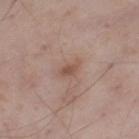Q: How was this image acquired?
A: total-body-photography crop, ~15 mm field of view
Q: Patient demographics?
A: male, in their mid-50s
Q: What is the lesion's diameter?
A: ≈3 mm
Q: What lighting was used for the tile?
A: white-light
Q: Automated lesion metrics?
A: a mean CIELAB color near L≈52 a*≈19 b*≈26, about 8 CIELAB-L* units darker than the surrounding skin, and a normalized border contrast of about 6.5; a classifier nevus-likeness of about 0/100 and lesion-presence confidence of about 100/100
Q: Lesion location?
A: the left thigh